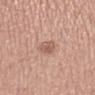<record>
  <biopsy_status>not biopsied; imaged during a skin examination</biopsy_status>
</record>